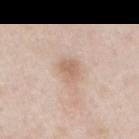Case summary:
– notes: no biopsy performed (imaged during a skin exam)
– diameter: about 3 mm
– image: ~15 mm crop, total-body skin-cancer survey
– site: the abdomen
– patient: male, in their 40s
– tile lighting: white-light illumination